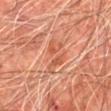Clinical impression: Imaged during a routine full-body skin examination; the lesion was not biopsied and no histopathology is available. Image and clinical context: A roughly 15 mm field-of-view crop from a total-body skin photograph. From the chest. The total-body-photography lesion software estimated an area of roughly 8 mm² and a shape-asymmetry score of about 0.45 (0 = symmetric). The analysis additionally found a lesion color around L≈51 a*≈27 b*≈33 in CIELAB, a lesion–skin lightness drop of about 7, and a lesion-to-skin contrast of about 5.5 (normalized; higher = more distinct). A male patient roughly 65 years of age. Longest diameter approximately 4.5 mm.